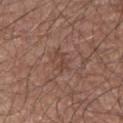Image and clinical context: The tile uses white-light illumination. This image is a 15 mm lesion crop taken from a total-body photograph. The recorded lesion diameter is about 2.5 mm. A male subject, roughly 75 years of age. On the leg. The lesion-visualizer software estimated about 6 CIELAB-L* units darker than the surrounding skin and a normalized lesion–skin contrast near 5.5.